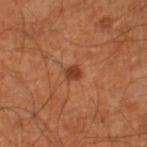Captured during whole-body skin photography for melanoma surveillance; the lesion was not biopsied.
Approximately 2 mm at its widest.
A male subject, about 60 years old.
This image is a 15 mm lesion crop taken from a total-body photograph.
This is a cross-polarized tile.
From the right lower leg.
The lesion-visualizer software estimated a border-irregularity index near 2/10, a within-lesion color-variation index near 1/10, and a peripheral color-asymmetry measure near 0.5. It also reported a classifier nevus-likeness of about 90/100.